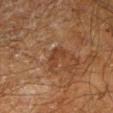Imaged during a routine full-body skin examination; the lesion was not biopsied and no histopathology is available.
A close-up tile cropped from a whole-body skin photograph, about 15 mm across.
The subject is a male approximately 60 years of age.
The tile uses cross-polarized illumination.
Located on the left lower leg.
The lesion's longest dimension is about 3 mm.